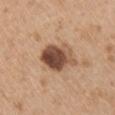Recorded during total-body skin imaging; not selected for excision or biopsy.
The lesion is on the left upper arm.
A male subject, aged around 50.
A lesion tile, about 15 mm wide, cut from a 3D total-body photograph.
Imaged with white-light lighting.
Automated image analysis of the tile measured a footprint of about 13 mm², an eccentricity of roughly 0.7, and two-axis asymmetry of about 0.25. The software also gave a classifier nevus-likeness of about 45/100 and a detector confidence of about 100 out of 100 that the crop contains a lesion.
The lesion's longest dimension is about 5 mm.